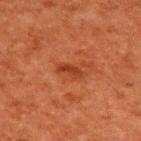Clinical impression:
Imaged during a routine full-body skin examination; the lesion was not biopsied and no histopathology is available.
Background:
Located on the upper back. A male patient aged 58 to 62. A region of skin cropped from a whole-body photographic capture, roughly 15 mm wide. Captured under cross-polarized illumination. The lesion's longest dimension is about 3 mm. Automated image analysis of the tile measured a lesion–skin lightness drop of about 7 and a normalized lesion–skin contrast near 7. The analysis additionally found border irregularity of about 3 on a 0–10 scale and a color-variation rating of about 0.5/10. And it measured an automated nevus-likeness rating near 5 out of 100 and a lesion-detection confidence of about 100/100.